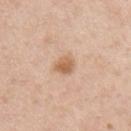Captured during whole-body skin photography for melanoma surveillance; the lesion was not biopsied. A male patient, aged 38–42. The recorded lesion diameter is about 2.5 mm. Cropped from a total-body skin-imaging series; the visible field is about 15 mm. This is a white-light tile. Automated tile analysis of the lesion measured a mean CIELAB color near L≈61 a*≈20 b*≈34 and a lesion–skin lightness drop of about 11. The lesion is located on the arm.